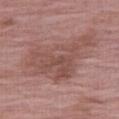Imaged during a routine full-body skin examination; the lesion was not biopsied and no histopathology is available. This is a white-light tile. From the right thigh. A region of skin cropped from a whole-body photographic capture, roughly 15 mm wide. A female patient, aged 63–67. An algorithmic analysis of the crop reported an outline eccentricity of about 0.8 (0 = round, 1 = elongated). It also reported a border-irregularity rating of about 6.5/10, a color-variation rating of about 4/10, and peripheral color asymmetry of about 1.5. And it measured a classifier nevus-likeness of about 0/100 and lesion-presence confidence of about 100/100.